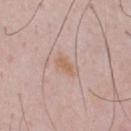Recorded during total-body skin imaging; not selected for excision or biopsy. The recorded lesion diameter is about 2.5 mm. Automated image analysis of the tile measured a lesion area of about 2.5 mm², an eccentricity of roughly 0.9, and a shape-asymmetry score of about 0.25 (0 = symmetric). It also reported an automated nevus-likeness rating near 10 out of 100 and a lesion-detection confidence of about 100/100. On the chest. The subject is a male aged approximately 50. The tile uses white-light illumination. This image is a 15 mm lesion crop taken from a total-body photograph.